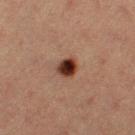| field | value |
|---|---|
| workup | catalogued during a skin exam; not biopsied |
| acquisition | total-body-photography crop, ~15 mm field of view |
| subject | female, roughly 40 years of age |
| location | the leg |